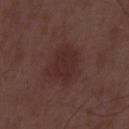notes = catalogued during a skin exam; not biopsied
subject = male, in their 50s
diameter = ~4.5 mm (longest diameter)
illumination = white-light
image source = 15 mm crop, total-body photography
automated metrics = an area of roughly 12 mm²; a border-irregularity rating of about 2.5/10 and a within-lesion color-variation index near 2/10